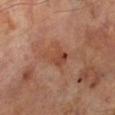follow-up: imaged on a skin check; not biopsied
lighting: cross-polarized
location: the left lower leg
acquisition: ~15 mm crop, total-body skin-cancer survey
subject: male, aged around 70
automated lesion analysis: an eccentricity of roughly 0.75 and a shape-asymmetry score of about 0.3 (0 = symmetric); an average lesion color of about L≈45 a*≈23 b*≈30 (CIELAB) and about 7 CIELAB-L* units darker than the surrounding skin; a border-irregularity index near 3.5/10, internal color variation of about 4.5 on a 0–10 scale, and a peripheral color-asymmetry measure near 1.5
size: ~3.5 mm (longest diameter)